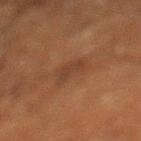Assessment:
Imaged during a routine full-body skin examination; the lesion was not biopsied and no histopathology is available.
Image and clinical context:
This is a cross-polarized tile. A male patient, aged 63 to 67. The lesion's longest dimension is about 2.5 mm. On the left lower leg. A 15 mm close-up extracted from a 3D total-body photography capture.